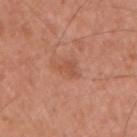Findings:
• notes: no biopsy performed (imaged during a skin exam)
• subject: male, aged around 55
• image source: total-body-photography crop, ~15 mm field of view
• lesion diameter: about 3 mm
• site: the right upper arm